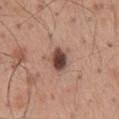This lesion was catalogued during total-body skin photography and was not selected for biopsy. The lesion's longest dimension is about 3 mm. A close-up tile cropped from a whole-body skin photograph, about 15 mm across. The lesion-visualizer software estimated a footprint of about 5.5 mm², a shape eccentricity near 0.7, and a shape-asymmetry score of about 0.15 (0 = symmetric). It also reported a mean CIELAB color near L≈44 a*≈20 b*≈23, a lesion–skin lightness drop of about 18, and a lesion-to-skin contrast of about 12.5 (normalized; higher = more distinct). The software also gave border irregularity of about 1.5 on a 0–10 scale. The lesion is on the mid back. Imaged with white-light lighting. The patient is a male aged 58–62.